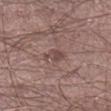biopsy_status: not biopsied; imaged during a skin examination
image:
  source: total-body photography crop
  field_of_view_mm: 15
site: left lower leg
patient:
  sex: male
  age_approx: 45
lesion_size:
  long_diameter_mm_approx: 2.5
automated_metrics:
  area_mm2_approx: 3.5
  eccentricity: 0.8
  nevus_likeness_0_100: 0